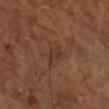{"biopsy_status": "not biopsied; imaged during a skin examination", "image": {"source": "total-body photography crop", "field_of_view_mm": 15}, "patient": {"sex": "male", "age_approx": 65}, "lesion_size": {"long_diameter_mm_approx": 3.0}, "site": "left forearm"}